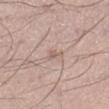Q: Is there a histopathology result?
A: no biopsy performed (imaged during a skin exam)